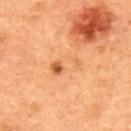| field | value |
|---|---|
| follow-up | total-body-photography surveillance lesion; no biopsy |
| patient | male, aged approximately 50 |
| body site | the upper back |
| image source | 15 mm crop, total-body photography |